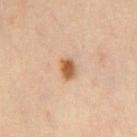Notes:
* biopsy status · catalogued during a skin exam; not biopsied
* site · the chest
* patient · male, aged approximately 45
* acquisition · ~15 mm crop, total-body skin-cancer survey